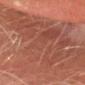Assessment:
Part of a total-body skin-imaging series; this lesion was reviewed on a skin check and was not flagged for biopsy.
Background:
Imaged with cross-polarized lighting. A 15 mm close-up tile from a total-body photography series done for melanoma screening. A male subject aged 68 to 72. The lesion's longest dimension is about 21.5 mm. The lesion is located on the head or neck.